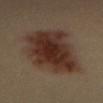This lesion was catalogued during total-body skin photography and was not selected for biopsy.
Located on the front of the torso.
The patient is a male in their 40s.
Cropped from a whole-body photographic skin survey; the tile spans about 15 mm.
The tile uses cross-polarized illumination.